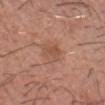Q: Is there a histopathology result?
A: imaged on a skin check; not biopsied
Q: Patient demographics?
A: male, aged 28 to 32
Q: Where on the body is the lesion?
A: the head or neck
Q: How was the tile lit?
A: white-light
Q: How was this image acquired?
A: 15 mm crop, total-body photography